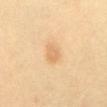biopsy status — total-body-photography surveillance lesion; no biopsy | image source — ~15 mm tile from a whole-body skin photo | tile lighting — cross-polarized | subject — female, roughly 40 years of age | automated lesion analysis — a mean CIELAB color near L≈71 a*≈20 b*≈41, roughly 8 lightness units darker than nearby skin, and a lesion-to-skin contrast of about 5 (normalized; higher = more distinct) | diameter — ≈2 mm | anatomic site — the abdomen.